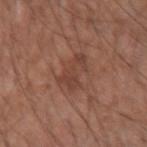Captured during whole-body skin photography for melanoma surveillance; the lesion was not biopsied. The lesion is on the arm. A roughly 15 mm field-of-view crop from a total-body skin photograph. Automated tile analysis of the lesion measured an average lesion color of about L≈42 a*≈21 b*≈26 (CIELAB), a lesion–skin lightness drop of about 7, and a lesion-to-skin contrast of about 6 (normalized; higher = more distinct). The patient is a male aged approximately 55. Approximately 4 mm at its widest.